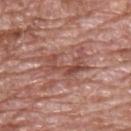No biopsy was performed on this lesion — it was imaged during a full skin examination and was not determined to be concerning. This is a white-light tile. From the upper back. Longest diameter approximately 6 mm. The subject is a male in their 70s. A 15 mm crop from a total-body photograph taken for skin-cancer surveillance.